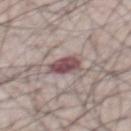The lesion was photographed on a routine skin check and not biopsied; there is no pathology result. This is a white-light tile. A 15 mm crop from a total-body photograph taken for skin-cancer surveillance. From the front of the torso. The lesion-visualizer software estimated a lesion color around L≈49 a*≈19 b*≈15 in CIELAB, roughly 14 lightness units darker than nearby skin, and a normalized border contrast of about 10. It also reported border irregularity of about 2.5 on a 0–10 scale, internal color variation of about 4.5 on a 0–10 scale, and peripheral color asymmetry of about 1.5. It also reported an automated nevus-likeness rating near 15 out of 100 and a lesion-detection confidence of about 80/100. The subject is a male about 65 years old.